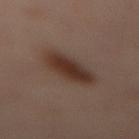<tbp_lesion>
<biopsy_status>not biopsied; imaged during a skin examination</biopsy_status>
<image>
  <source>total-body photography crop</source>
  <field_of_view_mm>15</field_of_view_mm>
</image>
<patient>
  <sex>female</sex>
  <age_approx>55</age_approx>
</patient>
<automated_metrics>
  <area_mm2_approx>12.0</area_mm2_approx>
  <eccentricity>0.95</eccentricity>
  <shape_asymmetry>0.15</shape_asymmetry>
  <cielab_L>26</cielab_L>
  <cielab_a>14</cielab_a>
  <cielab_b>20</cielab_b>
  <vs_skin_darker_L>9.0</vs_skin_darker_L>
  <vs_skin_contrast_norm>10.0</vs_skin_contrast_norm>
  <border_irregularity_0_10>2.5</border_irregularity_0_10>
  <peripheral_color_asymmetry>1.0</peripheral_color_asymmetry>
</automated_metrics>
<site>mid back</site>
<lighting>cross-polarized</lighting>
<lesion_size>
  <long_diameter_mm_approx>6.5</long_diameter_mm_approx>
</lesion_size>
</tbp_lesion>